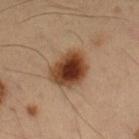Clinical impression: The lesion was photographed on a routine skin check and not biopsied; there is no pathology result. Clinical summary: The lesion is on the left forearm. A close-up tile cropped from a whole-body skin photograph, about 15 mm across. A male subject aged approximately 55. The lesion's longest dimension is about 4.5 mm. Automated tile analysis of the lesion measured border irregularity of about 2 on a 0–10 scale, internal color variation of about 6 on a 0–10 scale, and peripheral color asymmetry of about 1.5.